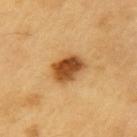Notes:
* biopsy status · total-body-photography surveillance lesion; no biopsy
* subject · male, aged around 60
* location · the arm
* imaging modality · 15 mm crop, total-body photography